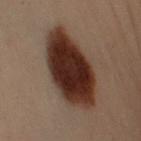Q: Was a biopsy performed?
A: catalogued during a skin exam; not biopsied
Q: Lesion size?
A: ≈9 mm
Q: What is the anatomic site?
A: the left arm
Q: What is the imaging modality?
A: total-body-photography crop, ~15 mm field of view
Q: How was the tile lit?
A: cross-polarized
Q: Who is the patient?
A: male, aged approximately 50